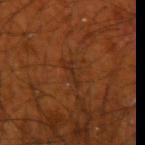Case summary:
* notes: catalogued during a skin exam; not biopsied
* body site: the left upper arm
* lesion diameter: ≈3 mm
* subject: male, aged 68–72
* imaging modality: total-body-photography crop, ~15 mm field of view
* tile lighting: cross-polarized